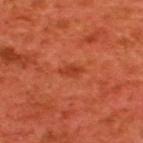No biopsy was performed on this lesion — it was imaged during a full skin examination and was not determined to be concerning.
A lesion tile, about 15 mm wide, cut from a 3D total-body photograph.
This is a cross-polarized tile.
From the back.
The patient is a male in their 60s.